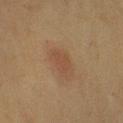<tbp_lesion>
<biopsy_status>not biopsied; imaged during a skin examination</biopsy_status>
<lighting>cross-polarized</lighting>
<image>
  <source>total-body photography crop</source>
  <field_of_view_mm>15</field_of_view_mm>
</image>
<lesion_size>
  <long_diameter_mm_approx>3.0</long_diameter_mm_approx>
</lesion_size>
<site>arm</site>
<patient>
  <sex>female</sex>
  <age_approx>55</age_approx>
</patient>
</tbp_lesion>